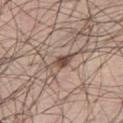biopsy status: no biopsy performed (imaged during a skin exam) | body site: the front of the torso | TBP lesion metrics: a lesion–skin lightness drop of about 12 and a normalized lesion–skin contrast near 8.5; a border-irregularity index near 4/10, a within-lesion color-variation index near 3/10, and radial color variation of about 0.5 | patient: male, about 70 years old | imaging modality: ~15 mm crop, total-body skin-cancer survey.